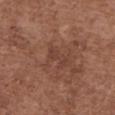Case summary:
* follow-up · no biopsy performed (imaged during a skin exam)
* body site · the upper back
* image source · 15 mm crop, total-body photography
* patient · female, aged 73–77